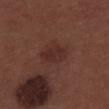  lesion_size:
    long_diameter_mm_approx: 3.5
  patient:
    sex: male
    age_approx: 30
  image:
    source: total-body photography crop
    field_of_view_mm: 15
  lighting: white-light
  site: upper back